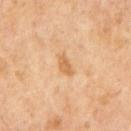This lesion was catalogued during total-body skin photography and was not selected for biopsy. A close-up tile cropped from a whole-body skin photograph, about 15 mm across. Longest diameter approximately 3 mm. Captured under cross-polarized illumination. A male subject aged approximately 65. The total-body-photography lesion software estimated a footprint of about 3.5 mm², an outline eccentricity of about 0.9 (0 = round, 1 = elongated), and a symmetry-axis asymmetry near 0.35. And it measured a border-irregularity index near 3.5/10, a color-variation rating of about 1/10, and radial color variation of about 0.5.